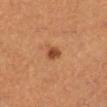Findings:
* biopsy status · catalogued during a skin exam; not biopsied
* image · total-body-photography crop, ~15 mm field of view
* automated lesion analysis · a lesion area of about 3.5 mm², an outline eccentricity of about 0.65 (0 = round, 1 = elongated), and a symmetry-axis asymmetry near 0.15; a lesion color around L≈43 a*≈24 b*≈34 in CIELAB and a lesion–skin lightness drop of about 11; a border-irregularity rating of about 1.5/10 and a within-lesion color-variation index near 2/10
* site · the left lower leg
* subject · female, in their 30s
* lighting · cross-polarized illumination